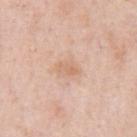<record>
<biopsy_status>not biopsied; imaged during a skin examination</biopsy_status>
<patient>
  <sex>male</sex>
  <age_approx>50</age_approx>
</patient>
<site>chest</site>
<lighting>white-light</lighting>
<image>
  <source>total-body photography crop</source>
  <field_of_view_mm>15</field_of_view_mm>
</image>
<lesion_size>
  <long_diameter_mm_approx>3.0</long_diameter_mm_approx>
</lesion_size>
</record>